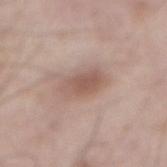Q: Is there a histopathology result?
A: catalogued during a skin exam; not biopsied
Q: What did automated image analysis measure?
A: a footprint of about 7.5 mm², a shape eccentricity near 0.75, and a shape-asymmetry score of about 0.2 (0 = symmetric); a border-irregularity index near 2.5/10, a within-lesion color-variation index near 2.5/10, and a peripheral color-asymmetry measure near 0.5; a nevus-likeness score of about 60/100 and a detector confidence of about 100 out of 100 that the crop contains a lesion
Q: Lesion location?
A: the abdomen
Q: What is the lesion's diameter?
A: ≈4 mm
Q: Patient demographics?
A: male, aged 53–57
Q: What lighting was used for the tile?
A: white-light illumination
Q: What kind of image is this?
A: 15 mm crop, total-body photography